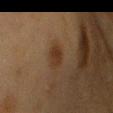| feature | finding |
|---|---|
| follow-up | no biopsy performed (imaged during a skin exam) |
| site | the front of the torso |
| lighting | cross-polarized |
| size | ~3 mm (longest diameter) |
| image source | 15 mm crop, total-body photography |
| patient | female, aged 53–57 |